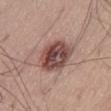Assessment:
The lesion was photographed on a routine skin check and not biopsied; there is no pathology result.
Clinical summary:
This is a white-light tile. Measured at roughly 4.5 mm in maximum diameter. A 15 mm close-up extracted from a 3D total-body photography capture. Automated image analysis of the tile measured an eccentricity of roughly 0.55 and a symmetry-axis asymmetry near 0.15. The analysis additionally found an average lesion color of about L≈46 a*≈21 b*≈22 (CIELAB), roughly 16 lightness units darker than nearby skin, and a normalized lesion–skin contrast near 11. The software also gave a border-irregularity index near 1.5/10, internal color variation of about 8.5 on a 0–10 scale, and radial color variation of about 3. The analysis additionally found a nevus-likeness score of about 40/100 and a detector confidence of about 100 out of 100 that the crop contains a lesion. The lesion is located on the abdomen. The subject is a male in their mid- to late 50s.